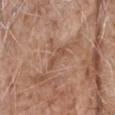Acquisition and patient details:
A male patient in their mid- to late 70s. The total-body-photography lesion software estimated a within-lesion color-variation index near 0.5/10 and radial color variation of about 0. The software also gave a nevus-likeness score of about 0/100 and a lesion-detection confidence of about 65/100. A region of skin cropped from a whole-body photographic capture, roughly 15 mm wide. From the head or neck.